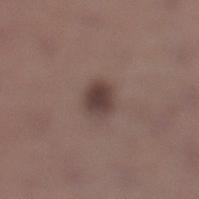Q: Is there a histopathology result?
A: total-body-photography surveillance lesion; no biopsy
Q: How was this image acquired?
A: ~15 mm tile from a whole-body skin photo
Q: Patient demographics?
A: male, about 45 years old
Q: Where on the body is the lesion?
A: the left lower leg
Q: What did automated image analysis measure?
A: a footprint of about 6 mm², an outline eccentricity of about 0.45 (0 = round, 1 = elongated), and a symmetry-axis asymmetry near 0.15; a mean CIELAB color near L≈40 a*≈15 b*≈19, a lesion–skin lightness drop of about 11, and a normalized lesion–skin contrast near 9